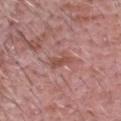Q: Was a biopsy performed?
A: no biopsy performed (imaged during a skin exam)
Q: Lesion size?
A: ~3 mm (longest diameter)
Q: Where on the body is the lesion?
A: the head or neck
Q: Patient demographics?
A: male, aged 58 to 62
Q: What kind of image is this?
A: 15 mm crop, total-body photography